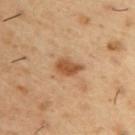Case summary:
• location · the upper back
• illumination · cross-polarized
• image · ~15 mm tile from a whole-body skin photo
• automated lesion analysis · an area of roughly 6 mm²; a mean CIELAB color near L≈52 a*≈21 b*≈36, a lesion–skin lightness drop of about 12, and a normalized lesion–skin contrast near 8.5; a border-irregularity index near 2.5/10 and a peripheral color-asymmetry measure near 1
• subject · male, in their mid- to late 50s